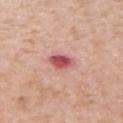workup = no biopsy performed (imaged during a skin exam)
location = the left upper arm
lesion diameter = about 3 mm
subject = male, aged around 60
image = ~15 mm tile from a whole-body skin photo
illumination = white-light